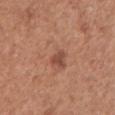The lesion was tiled from a total-body skin photograph and was not biopsied. A 15 mm close-up extracted from a 3D total-body photography capture. The lesion is located on the front of the torso. A male patient approximately 75 years of age. Approximately 4.5 mm at its widest.